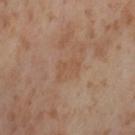biopsy status = no biopsy performed (imaged during a skin exam) | site = the leg | lesion size = ~3 mm (longest diameter) | image source = 15 mm crop, total-body photography | subject = female, in their mid-50s.